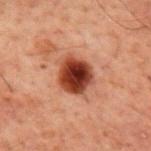Captured during whole-body skin photography for melanoma surveillance; the lesion was not biopsied.
The tile uses cross-polarized illumination.
An algorithmic analysis of the crop reported an area of roughly 12 mm². And it measured an automated nevus-likeness rating near 100 out of 100.
Located on the mid back.
Cropped from a whole-body photographic skin survey; the tile spans about 15 mm.
The patient is a male in their 60s.
About 4 mm across.